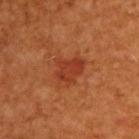Q: Is there a histopathology result?
A: imaged on a skin check; not biopsied
Q: Automated lesion metrics?
A: a mean CIELAB color near L≈36 a*≈30 b*≈34, about 8 CIELAB-L* units darker than the surrounding skin, and a normalized border contrast of about 6.5; a border-irregularity index near 3.5/10 and a peripheral color-asymmetry measure near 1; lesion-presence confidence of about 100/100
Q: Who is the patient?
A: male, aged approximately 60
Q: Lesion location?
A: the upper back
Q: Lesion size?
A: ≈3.5 mm
Q: How was this image acquired?
A: 15 mm crop, total-body photography